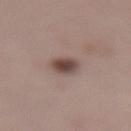Q: Was this lesion biopsied?
A: total-body-photography surveillance lesion; no biopsy
Q: What are the patient's age and sex?
A: female, aged approximately 45
Q: What kind of image is this?
A: 15 mm crop, total-body photography
Q: Automated lesion metrics?
A: a lesion–skin lightness drop of about 13 and a normalized border contrast of about 10
Q: Lesion size?
A: about 3 mm
Q: What is the anatomic site?
A: the lower back